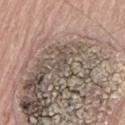Clinical impression: Imaged during a routine full-body skin examination; the lesion was not biopsied and no histopathology is available. Image and clinical context: Cropped from a total-body skin-imaging series; the visible field is about 15 mm. On the left thigh. This is a white-light tile. Automated tile analysis of the lesion measured an area of roughly 1 mm² and an eccentricity of roughly 0.7. It also reported a border-irregularity index near 2.5/10, a color-variation rating of about 0/10, and a peripheral color-asymmetry measure near 0. The software also gave a nevus-likeness score of about 0/100 and a lesion-detection confidence of about 0/100. A male patient aged around 75. About 1 mm across.